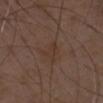{"biopsy_status": "not biopsied; imaged during a skin examination", "lighting": "white-light", "lesion_size": {"long_diameter_mm_approx": 3.5}, "patient": {"sex": "male", "age_approx": 50}, "image": {"source": "total-body photography crop", "field_of_view_mm": 15}}